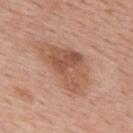<case>
  <biopsy_status>not biopsied; imaged during a skin examination</biopsy_status>
  <site>upper back</site>
  <lesion_size>
    <long_diameter_mm_approx>7.0</long_diameter_mm_approx>
  </lesion_size>
  <automated_metrics>
    <cielab_L>55</cielab_L>
    <cielab_a>22</cielab_a>
    <cielab_b>31</cielab_b>
    <vs_skin_darker_L>10.0</vs_skin_darker_L>
    <vs_skin_contrast_norm>7.0</vs_skin_contrast_norm>
    <border_irregularity_0_10>4.5</border_irregularity_0_10>
    <color_variation_0_10>5.0</color_variation_0_10>
  </automated_metrics>
  <image>
    <source>total-body photography crop</source>
    <field_of_view_mm>15</field_of_view_mm>
  </image>
  <lighting>white-light</lighting>
  <patient>
    <sex>male</sex>
    <age_approx>60</age_approx>
  </patient>
</case>